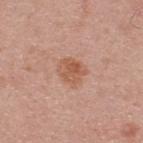This lesion was catalogued during total-body skin photography and was not selected for biopsy.
A male subject approximately 45 years of age.
An algorithmic analysis of the crop reported a lesion area of about 8 mm² and an eccentricity of roughly 0.45. It also reported a normalized border contrast of about 7. It also reported internal color variation of about 4 on a 0–10 scale and a peripheral color-asymmetry measure near 1.5.
The recorded lesion diameter is about 3.5 mm.
A close-up tile cropped from a whole-body skin photograph, about 15 mm across.
Captured under white-light illumination.
The lesion is located on the upper back.